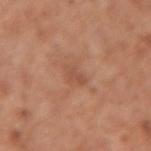notes: imaged on a skin check; not biopsied
automated metrics: a footprint of about 3.5 mm², a shape eccentricity near 0.8, and a shape-asymmetry score of about 0.3 (0 = symmetric); a border-irregularity index near 3/10, a color-variation rating of about 1.5/10, and peripheral color asymmetry of about 0.5
tile lighting: white-light
diameter: ~2.5 mm (longest diameter)
imaging modality: total-body-photography crop, ~15 mm field of view
patient: female, roughly 50 years of age
anatomic site: the left forearm